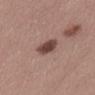The lesion was tiled from a total-body skin photograph and was not biopsied.